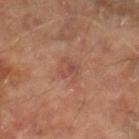Impression: Recorded during total-body skin imaging; not selected for excision or biopsy. Background: A 15 mm close-up extracted from a 3D total-body photography capture. The subject is a male aged 63 to 67. Located on the right lower leg. An algorithmic analysis of the crop reported a mean CIELAB color near L≈47 a*≈23 b*≈27 and a lesion–skin lightness drop of about 6. And it measured a border-irregularity index near 2.5/10, internal color variation of about 3.5 on a 0–10 scale, and a peripheral color-asymmetry measure near 1.